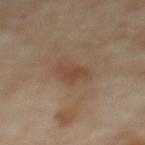<tbp_lesion>
  <biopsy_status>not biopsied; imaged during a skin examination</biopsy_status>
  <image>
    <source>total-body photography crop</source>
    <field_of_view_mm>15</field_of_view_mm>
  </image>
  <site>upper back</site>
  <lesion_size>
    <long_diameter_mm_approx>3.5</long_diameter_mm_approx>
  </lesion_size>
  <patient>
    <sex>female</sex>
    <age_approx>60</age_approx>
  </patient>
</tbp_lesion>